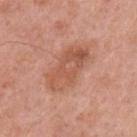Impression:
Part of a total-body skin-imaging series; this lesion was reviewed on a skin check and was not flagged for biopsy.
Context:
About 6 mm across. The patient is a male aged 53–57. An algorithmic analysis of the crop reported a lesion area of about 16 mm² and an outline eccentricity of about 0.85 (0 = round, 1 = elongated). The software also gave an average lesion color of about L≈56 a*≈25 b*≈32 (CIELAB). The analysis additionally found a border-irregularity rating of about 3/10 and peripheral color asymmetry of about 2. The analysis additionally found a nevus-likeness score of about 5/100 and lesion-presence confidence of about 100/100. A close-up tile cropped from a whole-body skin photograph, about 15 mm across. The tile uses white-light illumination. The lesion is on the left upper arm.